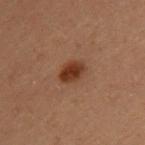Impression:
The lesion was tiled from a total-body skin photograph and was not biopsied.
Clinical summary:
The patient is a female aged around 40. Measured at roughly 3 mm in maximum diameter. Imaged with cross-polarized lighting. A lesion tile, about 15 mm wide, cut from a 3D total-body photograph. On the upper back.